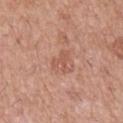Notes:
• workup: imaged on a skin check; not biopsied
• image: ~15 mm crop, total-body skin-cancer survey
• illumination: white-light illumination
• patient: male, aged around 55
• site: the right upper arm
• image-analysis metrics: a nevus-likeness score of about 0/100 and a detector confidence of about 100 out of 100 that the crop contains a lesion